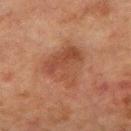Impression: Imaged during a routine full-body skin examination; the lesion was not biopsied and no histopathology is available. Acquisition and patient details: The tile uses cross-polarized illumination. The subject is a male in their mid- to late 60s. A roughly 15 mm field-of-view crop from a total-body skin photograph. The lesion is located on the chest.